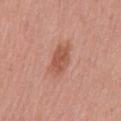Notes:
• subject · male, about 70 years old
• image-analysis metrics · border irregularity of about 2 on a 0–10 scale and a peripheral color-asymmetry measure near 1
• lighting · white-light
• location · the mid back
• acquisition · ~15 mm crop, total-body skin-cancer survey
• diameter · about 4 mm